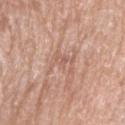Image and clinical context: The total-body-photography lesion software estimated a lesion area of about 5 mm², a shape eccentricity near 0.8, and a symmetry-axis asymmetry near 0.45. The analysis additionally found an average lesion color of about L≈59 a*≈21 b*≈28 (CIELAB), about 7 CIELAB-L* units darker than the surrounding skin, and a lesion-to-skin contrast of about 4.5 (normalized; higher = more distinct). And it measured border irregularity of about 8.5 on a 0–10 scale and radial color variation of about 0.5. And it measured a classifier nevus-likeness of about 0/100 and a lesion-detection confidence of about 65/100. A male patient, aged approximately 65. Longest diameter approximately 3.5 mm. Imaged with white-light lighting. Cropped from a whole-body photographic skin survey; the tile spans about 15 mm. Located on the right upper arm.